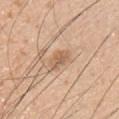<lesion>
  <biopsy_status>not biopsied; imaged during a skin examination</biopsy_status>
  <automated_metrics>
    <peripheral_color_asymmetry>1.0</peripheral_color_asymmetry>
    <lesion_detection_confidence_0_100>100</lesion_detection_confidence_0_100>
  </automated_metrics>
  <patient>
    <sex>male</sex>
    <age_approx>45</age_approx>
  </patient>
  <site>front of the torso</site>
  <lighting>white-light</lighting>
  <image>
    <source>total-body photography crop</source>
    <field_of_view_mm>15</field_of_view_mm>
  </image>
  <lesion_size>
    <long_diameter_mm_approx>3.0</long_diameter_mm_approx>
  </lesion_size>
</lesion>